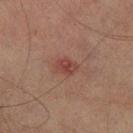<record>
<automated_metrics>
  <area_mm2_approx>3.5</area_mm2_approx>
  <shape_asymmetry>0.2</shape_asymmetry>
  <cielab_L>35</cielab_L>
  <cielab_a>22</cielab_a>
  <cielab_b>21</cielab_b>
  <vs_skin_darker_L>7.0</vs_skin_darker_L>
  <vs_skin_contrast_norm>6.5</vs_skin_contrast_norm>
  <border_irregularity_0_10>1.5</border_irregularity_0_10>
  <nevus_likeness_0_100>60</nevus_likeness_0_100>
</automated_metrics>
<lighting>cross-polarized</lighting>
<site>right lower leg</site>
<image>
  <source>total-body photography crop</source>
  <field_of_view_mm>15</field_of_view_mm>
</image>
<patient>
  <sex>male</sex>
  <age_approx>75</age_approx>
</patient>
</record>